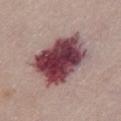biopsy status = no biopsy performed (imaged during a skin exam) | site = the chest | image source = 15 mm crop, total-body photography | patient = female, roughly 65 years of age.